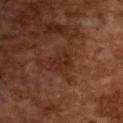<case>
<biopsy_status>not biopsied; imaged during a skin examination</biopsy_status>
<site>upper back</site>
<patient>
  <sex>female</sex>
  <age_approx>60</age_approx>
</patient>
<automated_metrics>
  <area_mm2_approx>3.5</area_mm2_approx>
  <eccentricity>0.8</eccentricity>
  <shape_asymmetry>0.5</shape_asymmetry>
  <cielab_L>23</cielab_L>
  <cielab_a>18</cielab_a>
  <cielab_b>25</cielab_b>
  <vs_skin_contrast_norm>6.0</vs_skin_contrast_norm>
</automated_metrics>
<lesion_size>
  <long_diameter_mm_approx>3.0</long_diameter_mm_approx>
</lesion_size>
<lighting>cross-polarized</lighting>
<image>
  <source>total-body photography crop</source>
  <field_of_view_mm>15</field_of_view_mm>
</image>
</case>